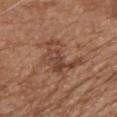workup = no biopsy performed (imaged during a skin exam) | subject = male, aged around 70 | tile lighting = white-light | anatomic site = the upper back | image = ~15 mm tile from a whole-body skin photo.